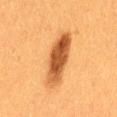workup: total-body-photography surveillance lesion; no biopsy
anatomic site: the lower back
image source: 15 mm crop, total-body photography
patient: female, aged 38–42
lighting: cross-polarized illumination